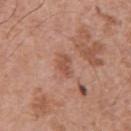workup: total-body-photography surveillance lesion; no biopsy